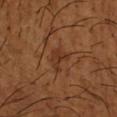<tbp_lesion>
<biopsy_status>not biopsied; imaged during a skin examination</biopsy_status>
<lesion_size>
  <long_diameter_mm_approx>3.0</long_diameter_mm_approx>
</lesion_size>
<site>left forearm</site>
<image>
  <source>total-body photography crop</source>
  <field_of_view_mm>15</field_of_view_mm>
</image>
<automated_metrics>
  <cielab_L>34</cielab_L>
  <cielab_a>22</cielab_a>
  <cielab_b>31</cielab_b>
  <vs_skin_darker_L>7.0</vs_skin_darker_L>
  <color_variation_0_10>1.5</color_variation_0_10>
  <peripheral_color_asymmetry>0.5</peripheral_color_asymmetry>
</automated_metrics>
<patient>
  <sex>male</sex>
  <age_approx>65</age_approx>
</patient>
<lighting>cross-polarized</lighting>
</tbp_lesion>